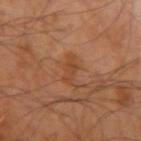The lesion was photographed on a routine skin check and not biopsied; there is no pathology result. An algorithmic analysis of the crop reported a lesion color around L≈44 a*≈23 b*≈34 in CIELAB, a lesion–skin lightness drop of about 6, and a lesion-to-skin contrast of about 5.5 (normalized; higher = more distinct). And it measured a border-irregularity rating of about 5.5/10, a within-lesion color-variation index near 1.5/10, and radial color variation of about 0.5. The lesion is on the left arm. The patient is a male about 50 years old. Imaged with cross-polarized lighting. A region of skin cropped from a whole-body photographic capture, roughly 15 mm wide.